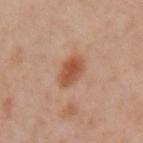Case summary:
– acquisition: ~15 mm tile from a whole-body skin photo
– automated metrics: two-axis asymmetry of about 0.2; a lesion–skin lightness drop of about 10 and a lesion-to-skin contrast of about 8 (normalized; higher = more distinct); a border-irregularity index near 1.5/10, a color-variation rating of about 4.5/10, and radial color variation of about 1.5
– anatomic site: the left upper arm
– subject: female, approximately 40 years of age
– illumination: cross-polarized